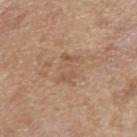The lesion was photographed on a routine skin check and not biopsied; there is no pathology result. The lesion is on the right forearm. Automated tile analysis of the lesion measured a lesion area of about 7 mm² and a shape-asymmetry score of about 0.4 (0 = symmetric). It also reported an average lesion color of about L≈55 a*≈18 b*≈31 (CIELAB) and a lesion–skin lightness drop of about 6. And it measured a border-irregularity rating of about 7.5/10, internal color variation of about 2.5 on a 0–10 scale, and radial color variation of about 1. The analysis additionally found an automated nevus-likeness rating near 0 out of 100 and a detector confidence of about 100 out of 100 that the crop contains a lesion. About 3.5 mm across. The tile uses white-light illumination. A lesion tile, about 15 mm wide, cut from a 3D total-body photograph. A female subject, roughly 65 years of age.